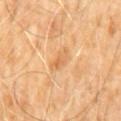biopsy status: imaged on a skin check; not biopsied
image-analysis metrics: a lesion color around L≈64 a*≈22 b*≈42 in CIELAB, about 8 CIELAB-L* units darker than the surrounding skin, and a normalized lesion–skin contrast near 5.5; border irregularity of about 4 on a 0–10 scale, a color-variation rating of about 1.5/10, and a peripheral color-asymmetry measure near 0.5
anatomic site: the abdomen
illumination: cross-polarized illumination
lesion size: about 4 mm
image source: total-body-photography crop, ~15 mm field of view
subject: male, roughly 60 years of age